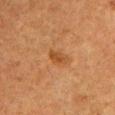The lesion was photographed on a routine skin check and not biopsied; there is no pathology result.
A 15 mm close-up tile from a total-body photography series done for melanoma screening.
A male patient, in their 50s.
The total-body-photography lesion software estimated an area of roughly 3.5 mm² and two-axis asymmetry of about 0.2. It also reported a border-irregularity rating of about 2/10. The software also gave a classifier nevus-likeness of about 10/100 and a detector confidence of about 100 out of 100 that the crop contains a lesion.
The recorded lesion diameter is about 2.5 mm.
Located on the left upper arm.
Captured under cross-polarized illumination.